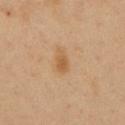- biopsy status: catalogued during a skin exam; not biopsied
- illumination: cross-polarized illumination
- size: ≈3 mm
- subject: male, roughly 50 years of age
- acquisition: 15 mm crop, total-body photography
- anatomic site: the chest
- TBP lesion metrics: an average lesion color of about L≈56 a*≈19 b*≈38 (CIELAB) and roughly 8 lightness units darker than nearby skin; border irregularity of about 3 on a 0–10 scale, internal color variation of about 2 on a 0–10 scale, and peripheral color asymmetry of about 0.5; a nevus-likeness score of about 65/100 and a lesion-detection confidence of about 100/100